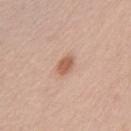Imaged during a routine full-body skin examination; the lesion was not biopsied and no histopathology is available. Cropped from a whole-body photographic skin survey; the tile spans about 15 mm. The subject is a female aged 58 to 62. From the chest. The lesion-visualizer software estimated a lesion color around L≈59 a*≈22 b*≈30 in CIELAB, roughly 11 lightness units darker than nearby skin, and a lesion-to-skin contrast of about 7.5 (normalized; higher = more distinct).